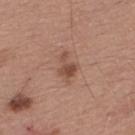follow-up: total-body-photography surveillance lesion; no biopsy
automated metrics: an area of roughly 6 mm² and an outline eccentricity of about 0.75 (0 = round, 1 = elongated); a lesion color around L≈49 a*≈21 b*≈28 in CIELAB and a normalized border contrast of about 7; a nevus-likeness score of about 65/100 and lesion-presence confidence of about 100/100
subject: male, approximately 55 years of age
image: ~15 mm tile from a whole-body skin photo
lesion diameter: ≈4 mm
anatomic site: the upper back